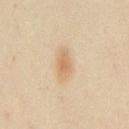No biopsy was performed on this lesion — it was imaged during a full skin examination and was not determined to be concerning. The tile uses cross-polarized illumination. A 15 mm crop from a total-body photograph taken for skin-cancer surveillance. The recorded lesion diameter is about 3.5 mm. From the chest. A male subject aged approximately 50. An algorithmic analysis of the crop reported a footprint of about 6.5 mm² and an outline eccentricity of about 0.8 (0 = round, 1 = elongated). It also reported a border-irregularity index near 2/10, a color-variation rating of about 2.5/10, and radial color variation of about 0.5.